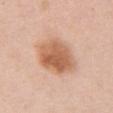Q: Was a biopsy performed?
A: catalogued during a skin exam; not biopsied
Q: What kind of image is this?
A: 15 mm crop, total-body photography
Q: How was the tile lit?
A: white-light illumination
Q: What are the patient's age and sex?
A: female, aged 48–52
Q: Where on the body is the lesion?
A: the front of the torso
Q: How large is the lesion?
A: about 6 mm